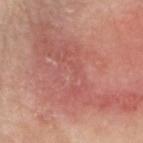Captured during whole-body skin photography for melanoma surveillance; the lesion was not biopsied.
The lesion is on the head or neck.
The lesion-visualizer software estimated a mean CIELAB color near L≈56 a*≈27 b*≈26, roughly 8 lightness units darker than nearby skin, and a lesion-to-skin contrast of about 5.5 (normalized; higher = more distinct). The software also gave border irregularity of about 9.5 on a 0–10 scale, a color-variation rating of about 5.5/10, and a peripheral color-asymmetry measure near 2. It also reported a nevus-likeness score of about 0/100 and a lesion-detection confidence of about 65/100.
Imaged with white-light lighting.
A close-up tile cropped from a whole-body skin photograph, about 15 mm across.
A male subject roughly 80 years of age.